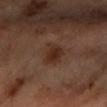This lesion was catalogued during total-body skin photography and was not selected for biopsy. The lesion-visualizer software estimated an eccentricity of roughly 0.65 and a shape-asymmetry score of about 0.25 (0 = symmetric). The software also gave an average lesion color of about L≈30 a*≈18 b*≈26 (CIELAB), roughly 8 lightness units darker than nearby skin, and a lesion-to-skin contrast of about 8 (normalized; higher = more distinct). And it measured a border-irregularity index near 2.5/10 and a within-lesion color-variation index near 3/10. And it measured an automated nevus-likeness rating near 60 out of 100 and a detector confidence of about 100 out of 100 that the crop contains a lesion. The lesion is located on the left forearm. A 15 mm crop from a total-body photograph taken for skin-cancer surveillance. A female subject aged 58–62. Longest diameter approximately 3.5 mm.